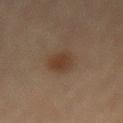The lesion was tiled from a total-body skin photograph and was not biopsied. Located on the mid back. The subject is a female aged 78 to 82. Cropped from a total-body skin-imaging series; the visible field is about 15 mm.